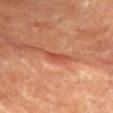– biopsy status: no biopsy performed (imaged during a skin exam)
– lesion size: about 3 mm
– patient: female, in their 80s
– image source: total-body-photography crop, ~15 mm field of view
– tile lighting: cross-polarized
– body site: the chest